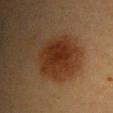Imaged during a routine full-body skin examination; the lesion was not biopsied and no histopathology is available. The patient is a female about 40 years old. Approximately 5 mm at its widest. This image is a 15 mm lesion crop taken from a total-body photograph. The lesion is on the upper back.